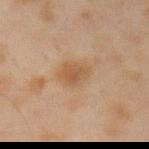site: left forearm
image:
  source: total-body photography crop
  field_of_view_mm: 15
patient:
  sex: male
  age_approx: 45
lesion_size:
  long_diameter_mm_approx: 3.5
automated_metrics:
  area_mm2_approx: 7.0
  eccentricity: 0.65
  shape_asymmetry: 0.2
  cielab_L: 44
  cielab_a: 16
  cielab_b: 29
  vs_skin_darker_L: 6.0
  vs_skin_contrast_norm: 6.0
  border_irregularity_0_10: 2.0
  color_variation_0_10: 2.5
  nevus_likeness_0_100: 70
  lesion_detection_confidence_0_100: 100